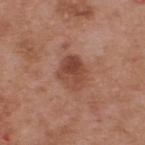Clinical impression: The lesion was photographed on a routine skin check and not biopsied; there is no pathology result. Context: The lesion's longest dimension is about 3.5 mm. From the upper back. A male patient, in their mid-50s. Automated image analysis of the tile measured a border-irregularity index near 2/10, a within-lesion color-variation index near 6/10, and peripheral color asymmetry of about 2.5. Cropped from a whole-body photographic skin survey; the tile spans about 15 mm. The tile uses white-light illumination.